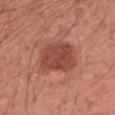Captured during whole-body skin photography for melanoma surveillance; the lesion was not biopsied. The lesion is on the arm. A male patient, in their 60s. An algorithmic analysis of the crop reported an area of roughly 15 mm² and a shape-asymmetry score of about 0.15 (0 = symmetric). It also reported roughly 11 lightness units darker than nearby skin and a normalized border contrast of about 8. Measured at roughly 5 mm in maximum diameter. A region of skin cropped from a whole-body photographic capture, roughly 15 mm wide. The tile uses white-light illumination.